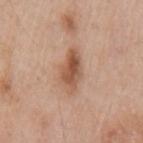Findings:
* workup: total-body-photography surveillance lesion; no biopsy
* location: the left upper arm
* diameter: ≈5 mm
* subject: male, aged 58–62
* image source: total-body-photography crop, ~15 mm field of view